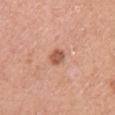Q: What did automated image analysis measure?
A: a lesion area of about 3.5 mm², an eccentricity of roughly 0.7, and two-axis asymmetry of about 0.2; a normalized lesion–skin contrast near 8; border irregularity of about 2 on a 0–10 scale, a color-variation rating of about 2/10, and radial color variation of about 1; a classifier nevus-likeness of about 75/100 and a lesion-detection confidence of about 100/100
Q: Who is the patient?
A: female, in their 40s
Q: Where on the body is the lesion?
A: the arm
Q: How large is the lesion?
A: ≈2.5 mm
Q: What is the imaging modality?
A: ~15 mm crop, total-body skin-cancer survey